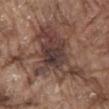The lesion was tiled from a total-body skin photograph and was not biopsied. The tile uses white-light illumination. A roughly 15 mm field-of-view crop from a total-body skin photograph. The lesion is on the abdomen. A male subject, aged 78 to 82. The lesion's longest dimension is about 10 mm.